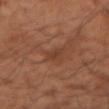• biopsy status — imaged on a skin check; not biopsied
• location — the arm
• image source — ~15 mm crop, total-body skin-cancer survey
• subject — male, aged around 55